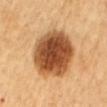Recorded during total-body skin imaging; not selected for excision or biopsy.